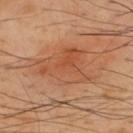follow-up=catalogued during a skin exam; not biopsied | lighting=cross-polarized | patient=male, in their 50s | location=the upper back | imaging modality=~15 mm crop, total-body skin-cancer survey | size=~7 mm (longest diameter).